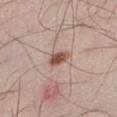Captured during whole-body skin photography for melanoma surveillance; the lesion was not biopsied. The patient is a male in their mid- to late 30s. A lesion tile, about 15 mm wide, cut from a 3D total-body photograph. The tile uses white-light illumination. The lesion is on the left lower leg. Longest diameter approximately 2.5 mm.